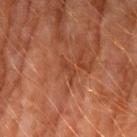  biopsy_status: not biopsied; imaged during a skin examination
  image:
    source: total-body photography crop
    field_of_view_mm: 15
  patient:
    sex: male
    age_approx: 60
  automated_metrics:
    area_mm2_approx: 2.5
    eccentricity: 0.9
    cielab_L: 40
    cielab_a: 26
    cielab_b: 32
    vs_skin_darker_L: 6.0
    vs_skin_contrast_norm: 5.0
    border_irregularity_0_10: 3.5
    peripheral_color_asymmetry: 0.0
    nevus_likeness_0_100: 0
  lesion_size:
    long_diameter_mm_approx: 2.5
  site: arm
  lighting: cross-polarized